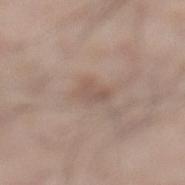  biopsy_status: not biopsied; imaged during a skin examination
  lighting: white-light
  image:
    source: total-body photography crop
    field_of_view_mm: 15
  patient:
    sex: male
    age_approx: 35
  site: leg
  lesion_size:
    long_diameter_mm_approx: 3.0